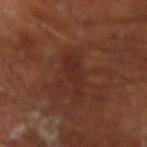| field | value |
|---|---|
| lesion diameter | ~5 mm (longest diameter) |
| image-analysis metrics | a symmetry-axis asymmetry near 0.35; a border-irregularity rating of about 4.5/10; a classifier nevus-likeness of about 0/100 and a lesion-detection confidence of about 95/100 |
| image | ~15 mm crop, total-body skin-cancer survey |
| body site | the right forearm |
| subject | male, in their mid-60s |